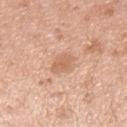notes = no biopsy performed (imaged during a skin exam)